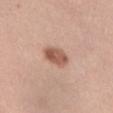Impression: The lesion was tiled from a total-body skin photograph and was not biopsied. Background: The lesion is on the abdomen. Automated tile analysis of the lesion measured a border-irregularity index near 2/10 and a within-lesion color-variation index near 4/10. Cropped from a total-body skin-imaging series; the visible field is about 15 mm. A female patient, in their 40s.